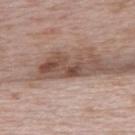Notes:
- biopsy status — catalogued during a skin exam; not biopsied
- imaging modality — total-body-photography crop, ~15 mm field of view
- subject — female, approximately 65 years of age
- site — the back